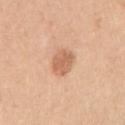* biopsy status: total-body-photography surveillance lesion; no biopsy
* patient: female, about 40 years old
* site: the right upper arm
* imaging modality: 15 mm crop, total-body photography
* lesion size: about 3 mm
* illumination: white-light illumination